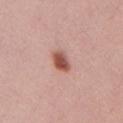follow-up = imaged on a skin check; not biopsied
subject = female, aged 23 to 27
tile lighting = white-light
image-analysis metrics = a mean CIELAB color near L≈53 a*≈26 b*≈27, roughly 15 lightness units darker than nearby skin, and a normalized lesion–skin contrast near 10.5
image = ~15 mm tile from a whole-body skin photo
size = about 2.5 mm
anatomic site = the chest